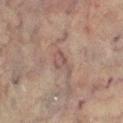• follow-up — no biopsy performed (imaged during a skin exam)
• image — total-body-photography crop, ~15 mm field of view
• patient — female, about 80 years old
• location — the left lower leg
• automated lesion analysis — a classifier nevus-likeness of about 0/100